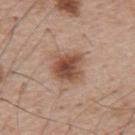{"biopsy_status": "not biopsied; imaged during a skin examination", "patient": {"sex": "male", "age_approx": 55}, "lesion_size": {"long_diameter_mm_approx": 4.0}, "automated_metrics": {"eccentricity": 0.5, "shape_asymmetry": 0.15, "cielab_L": 51, "cielab_a": 20, "cielab_b": 29, "vs_skin_contrast_norm": 9.0, "border_irregularity_0_10": 2.0, "peripheral_color_asymmetry": 1.5}, "image": {"source": "total-body photography crop", "field_of_view_mm": 15}}